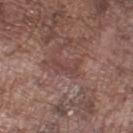This lesion was catalogued during total-body skin photography and was not selected for biopsy. A male patient approximately 75 years of age. A 15 mm crop from a total-body photograph taken for skin-cancer surveillance. About 3.5 mm across. The lesion is on the left lower leg. This is a white-light tile.